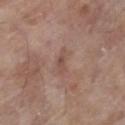The lesion was tiled from a total-body skin photograph and was not biopsied.
Cropped from a total-body skin-imaging series; the visible field is about 15 mm.
A male patient, aged around 80.
On the right lower leg.
Automated tile analysis of the lesion measured an area of roughly 3.5 mm² and an eccentricity of roughly 0.9. The analysis additionally found about 7 CIELAB-L* units darker than the surrounding skin and a normalized border contrast of about 5.5. The analysis additionally found border irregularity of about 3.5 on a 0–10 scale and a within-lesion color-variation index near 2.5/10. The analysis additionally found a nevus-likeness score of about 0/100 and lesion-presence confidence of about 100/100.